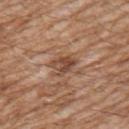notes — imaged on a skin check; not biopsied | acquisition — 15 mm crop, total-body photography | body site — the right upper arm | patient — male, aged around 60.